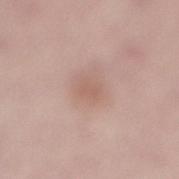Case summary:
* workup — total-body-photography surveillance lesion; no biopsy
* acquisition — 15 mm crop, total-body photography
* size — about 2.5 mm
* site — the lower back
* patient — male, in their mid-50s
* lighting — white-light
* TBP lesion metrics — an outline eccentricity of about 0.6 (0 = round, 1 = elongated); border irregularity of about 2.5 on a 0–10 scale and internal color variation of about 1.5 on a 0–10 scale; a nevus-likeness score of about 5/100